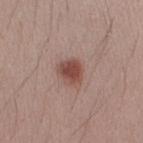Background: On the right forearm. Imaged with white-light lighting. Longest diameter approximately 3 mm. Automated image analysis of the tile measured about 12 CIELAB-L* units darker than the surrounding skin and a lesion-to-skin contrast of about 9 (normalized; higher = more distinct). The software also gave an automated nevus-likeness rating near 100 out of 100. A lesion tile, about 15 mm wide, cut from a 3D total-body photograph. A male patient about 40 years old.